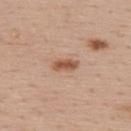The lesion was tiled from a total-body skin photograph and was not biopsied. A male patient aged approximately 55. The lesion is located on the upper back. This is a white-light tile. Measured at roughly 3.5 mm in maximum diameter. Cropped from a whole-body photographic skin survey; the tile spans about 15 mm. An algorithmic analysis of the crop reported a footprint of about 4 mm². The analysis additionally found about 12 CIELAB-L* units darker than the surrounding skin and a normalized border contrast of about 8.5. The software also gave a nevus-likeness score of about 100/100.